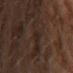workup = catalogued during a skin exam; not biopsied
image source = ~15 mm tile from a whole-body skin photo
automated metrics = a classifier nevus-likeness of about 0/100
anatomic site = the head or neck
subject = female, approximately 60 years of age
tile lighting = cross-polarized illumination